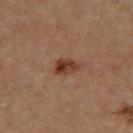* follow-up: total-body-photography surveillance lesion; no biopsy
* image: total-body-photography crop, ~15 mm field of view
* tile lighting: cross-polarized illumination
* subject: male, aged approximately 65
* body site: the left thigh
* automated lesion analysis: a lesion color around L≈39 a*≈22 b*≈30 in CIELAB, a lesion–skin lightness drop of about 11, and a normalized lesion–skin contrast near 9.5; a border-irregularity rating of about 2.5/10 and radial color variation of about 2.5
* lesion diameter: ~3 mm (longest diameter)